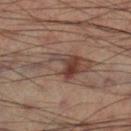Impression:
The lesion was photographed on a routine skin check and not biopsied; there is no pathology result.
Context:
The lesion is on the right lower leg. Approximately 8 mm at its widest. Captured under cross-polarized illumination. A male patient, about 50 years old. Cropped from a total-body skin-imaging series; the visible field is about 15 mm.